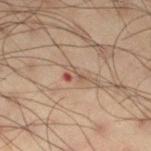No biopsy was performed on this lesion — it was imaged during a full skin examination and was not determined to be concerning.
A male patient, aged 48–52.
A lesion tile, about 15 mm wide, cut from a 3D total-body photograph.
The total-body-photography lesion software estimated a lesion area of about 3.5 mm², an outline eccentricity of about 0.8 (0 = round, 1 = elongated), and a symmetry-axis asymmetry near 0.5. And it measured a lesion color around L≈40 a*≈15 b*≈22 in CIELAB and a lesion-to-skin contrast of about 6 (normalized; higher = more distinct).
The lesion is located on the right thigh.